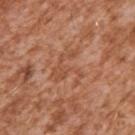The lesion was photographed on a routine skin check and not biopsied; there is no pathology result.
Captured under white-light illumination.
Cropped from a whole-body photographic skin survey; the tile spans about 15 mm.
The subject is a male roughly 45 years of age.
On the right upper arm.